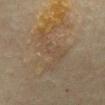Clinical impression: Captured during whole-body skin photography for melanoma surveillance; the lesion was not biopsied. Background: A male subject about 75 years old. Measured at roughly 7 mm in maximum diameter. The total-body-photography lesion software estimated a lesion color around L≈39 a*≈11 b*≈24 in CIELAB, roughly 4 lightness units darker than nearby skin, and a lesion-to-skin contrast of about 4 (normalized; higher = more distinct). And it measured a classifier nevus-likeness of about 0/100 and lesion-presence confidence of about 15/100. The lesion is located on the abdomen. A roughly 15 mm field-of-view crop from a total-body skin photograph. Captured under cross-polarized illumination.